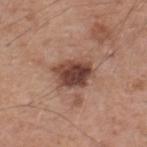Recorded during total-body skin imaging; not selected for excision or biopsy. Approximately 4.5 mm at its widest. From the upper back. Captured under white-light illumination. Automated image analysis of the tile measured a footprint of about 11 mm², a shape eccentricity near 0.7, and a shape-asymmetry score of about 0.25 (0 = symmetric). The software also gave a border-irregularity rating of about 3/10, a color-variation rating of about 6/10, and peripheral color asymmetry of about 2. And it measured an automated nevus-likeness rating near 80 out of 100 and lesion-presence confidence of about 100/100. A lesion tile, about 15 mm wide, cut from a 3D total-body photograph. A male subject in their mid-50s.